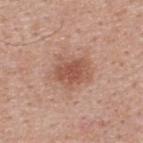biopsy status: catalogued during a skin exam; not biopsied
image-analysis metrics: a color-variation rating of about 3.5/10 and radial color variation of about 1
location: the upper back
subject: male, aged 38–42
tile lighting: white-light
image: ~15 mm tile from a whole-body skin photo
lesion diameter: about 5 mm